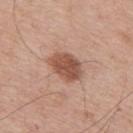No biopsy was performed on this lesion — it was imaged during a full skin examination and was not determined to be concerning. A male patient aged 63–67. An algorithmic analysis of the crop reported a lesion area of about 10 mm² and a symmetry-axis asymmetry near 0.15. The software also gave a classifier nevus-likeness of about 85/100 and lesion-presence confidence of about 100/100. From the mid back. A 15 mm close-up extracted from a 3D total-body photography capture. Imaged with white-light lighting. Approximately 4.5 mm at its widest.